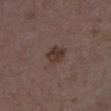The lesion was tiled from a total-body skin photograph and was not biopsied. The tile uses white-light illumination. A roughly 15 mm field-of-view crop from a total-body skin photograph. On the left lower leg. The lesion's longest dimension is about 3 mm. A female patient, aged 33 to 37.